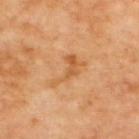Q: Was this lesion biopsied?
A: no biopsy performed (imaged during a skin exam)
Q: How large is the lesion?
A: about 4.5 mm
Q: What is the anatomic site?
A: the upper back
Q: What did automated image analysis measure?
A: an eccentricity of roughly 0.85 and a shape-asymmetry score of about 0.65 (0 = symmetric)
Q: Illumination type?
A: cross-polarized illumination
Q: Patient demographics?
A: roughly 65 years of age
Q: What is the imaging modality?
A: ~15 mm tile from a whole-body skin photo